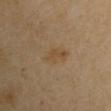| field | value |
|---|---|
| workup | imaged on a skin check; not biopsied |
| TBP lesion metrics | a lesion area of about 7 mm² and an eccentricity of roughly 0.85; border irregularity of about 2.5 on a 0–10 scale, a within-lesion color-variation index near 2.5/10, and peripheral color asymmetry of about 1 |
| site | the left upper arm |
| lesion size | about 4 mm |
| image | ~15 mm crop, total-body skin-cancer survey |
| patient | male, aged 68 to 72 |